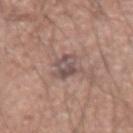Case summary:
* workup · catalogued during a skin exam; not biopsied
* automated lesion analysis · an area of roughly 7 mm² and a shape eccentricity near 0.55; a mean CIELAB color near L≈51 a*≈16 b*≈20, roughly 9 lightness units darker than nearby skin, and a normalized border contrast of about 7.5
* subject · male, approximately 55 years of age
* image source · ~15 mm crop, total-body skin-cancer survey
* lesion diameter · ≈3.5 mm
* tile lighting · white-light